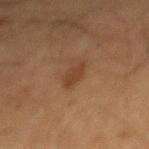No biopsy was performed on this lesion — it was imaged during a full skin examination and was not determined to be concerning. The lesion is located on the mid back. A male subject, aged approximately 65. Cropped from a total-body skin-imaging series; the visible field is about 15 mm. An algorithmic analysis of the crop reported a footprint of about 3.5 mm² and a shape-asymmetry score of about 0.3 (0 = symmetric). The software also gave a lesion color around L≈33 a*≈17 b*≈27 in CIELAB, about 7 CIELAB-L* units darker than the surrounding skin, and a lesion-to-skin contrast of about 6.5 (normalized; higher = more distinct). The analysis additionally found a border-irregularity rating of about 3.5/10, a within-lesion color-variation index near 1/10, and a peripheral color-asymmetry measure near 0.5. The software also gave a classifier nevus-likeness of about 65/100 and a detector confidence of about 100 out of 100 that the crop contains a lesion. Measured at roughly 3.5 mm in maximum diameter. Captured under cross-polarized illumination.